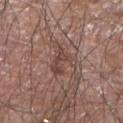Case summary:
* notes · imaged on a skin check; not biopsied
* TBP lesion metrics · an area of roughly 6.5 mm², a shape eccentricity near 0.7, and a symmetry-axis asymmetry near 0.6
* lighting · white-light
* patient · male, in their 80s
* size · ≈4 mm
* image source · ~15 mm tile from a whole-body skin photo
* anatomic site · the right forearm